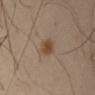The subject is a male aged approximately 40. Located on the right upper arm. Cropped from a whole-body photographic skin survey; the tile spans about 15 mm. The total-body-photography lesion software estimated an eccentricity of roughly 0.8 and a symmetry-axis asymmetry near 0.15. The software also gave a lesion color around L≈45 a*≈15 b*≈29 in CIELAB and about 10 CIELAB-L* units darker than the surrounding skin. And it measured an automated nevus-likeness rating near 95 out of 100 and a detector confidence of about 100 out of 100 that the crop contains a lesion.